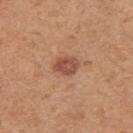The lesion was tiled from a total-body skin photograph and was not biopsied. Approximately 3 mm at its widest. The total-body-photography lesion software estimated about 11 CIELAB-L* units darker than the surrounding skin. It also reported radial color variation of about 1. And it measured a classifier nevus-likeness of about 65/100 and lesion-presence confidence of about 100/100. The lesion is located on the right upper arm. This image is a 15 mm lesion crop taken from a total-body photograph. A female patient aged approximately 30.